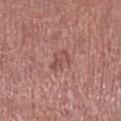Notes:
• location: the leg
• image: ~15 mm crop, total-body skin-cancer survey
• patient: male, approximately 65 years of age